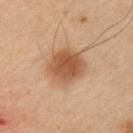Impression: No biopsy was performed on this lesion — it was imaged during a full skin examination and was not determined to be concerning. Image and clinical context: An algorithmic analysis of the crop reported a lesion area of about 14 mm², an outline eccentricity of about 0.35 (0 = round, 1 = elongated), and a shape-asymmetry score of about 0.1 (0 = symmetric). It also reported a lesion color around L≈56 a*≈22 b*≈36 in CIELAB, roughly 13 lightness units darker than nearby skin, and a lesion-to-skin contrast of about 8.5 (normalized; higher = more distinct). It also reported a border-irregularity index near 1/10, internal color variation of about 4.5 on a 0–10 scale, and a peripheral color-asymmetry measure near 1. Measured at roughly 4.5 mm in maximum diameter. This is a cross-polarized tile. From the left upper arm. A female subject about 40 years old. This image is a 15 mm lesion crop taken from a total-body photograph.